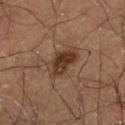Assessment: This lesion was catalogued during total-body skin photography and was not selected for biopsy. Clinical summary: Captured under cross-polarized illumination. The patient is a male approximately 60 years of age. The lesion is on the left thigh. Cropped from a total-body skin-imaging series; the visible field is about 15 mm. Longest diameter approximately 5 mm.